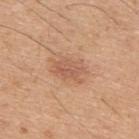Recorded during total-body skin imaging; not selected for excision or biopsy.
Automated image analysis of the tile measured a border-irregularity index near 3/10, a color-variation rating of about 3/10, and a peripheral color-asymmetry measure near 1. The software also gave a nevus-likeness score of about 45/100 and lesion-presence confidence of about 100/100.
Cropped from a whole-body photographic skin survey; the tile spans about 15 mm.
Located on the upper back.
The tile uses white-light illumination.
The lesion's longest dimension is about 3.5 mm.
A male patient roughly 45 years of age.